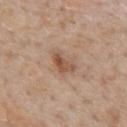Recorded during total-body skin imaging; not selected for excision or biopsy.
Cropped from a whole-body photographic skin survey; the tile spans about 15 mm.
This is a white-light tile.
A male subject, aged around 60.
The lesion is on the chest.